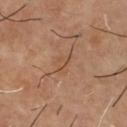{
  "biopsy_status": "not biopsied; imaged during a skin examination",
  "patient": {
    "sex": "male",
    "age_approx": 55
  },
  "site": "chest",
  "image": {
    "source": "total-body photography crop",
    "field_of_view_mm": 15
  },
  "lighting": "cross-polarized",
  "lesion_size": {
    "long_diameter_mm_approx": 3.0
  },
  "automated_metrics": {
    "cielab_L": 48,
    "cielab_a": 19,
    "cielab_b": 32,
    "vs_skin_darker_L": 5.0,
    "vs_skin_contrast_norm": 4.5
  }
}